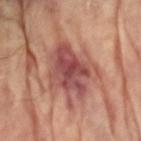workup: catalogued during a skin exam; not biopsied
lesion size: about 6.5 mm
location: the right arm
TBP lesion metrics: a footprint of about 22 mm², an outline eccentricity of about 0.75 (0 = round, 1 = elongated), and a symmetry-axis asymmetry near 0.35; a lesion color around L≈44 a*≈24 b*≈22 in CIELAB, about 11 CIELAB-L* units darker than the surrounding skin, and a normalized lesion–skin contrast near 9.5; a nevus-likeness score of about 0/100 and a lesion-detection confidence of about 85/100
image: ~15 mm tile from a whole-body skin photo
subject: female, about 80 years old